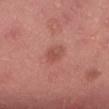Impression:
This lesion was catalogued during total-body skin photography and was not selected for biopsy.
Acquisition and patient details:
On the lower back. A male subject roughly 40 years of age. A region of skin cropped from a whole-body photographic capture, roughly 15 mm wide.